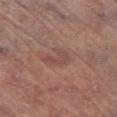  biopsy_status: not biopsied; imaged during a skin examination
  lesion_size:
    long_diameter_mm_approx: 3.5
  image:
    source: total-body photography crop
    field_of_view_mm: 15
  patient:
    sex: male
    age_approx: 70
  site: right lower leg